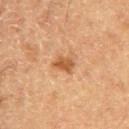workup: catalogued during a skin exam; not biopsied
subject: male, about 60 years old
size: ~2.5 mm (longest diameter)
lighting: cross-polarized
image source: ~15 mm tile from a whole-body skin photo